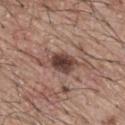Case summary:
* notes: catalogued during a skin exam; not biopsied
* location: the mid back
* illumination: white-light illumination
* imaging modality: total-body-photography crop, ~15 mm field of view
* patient: male, approximately 65 years of age
* TBP lesion metrics: internal color variation of about 5.5 on a 0–10 scale and peripheral color asymmetry of about 1; an automated nevus-likeness rating near 90 out of 100 and a detector confidence of about 100 out of 100 that the crop contains a lesion